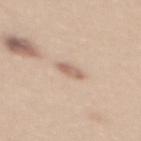Part of a total-body skin-imaging series; this lesion was reviewed on a skin check and was not flagged for biopsy.
On the upper back.
Captured under white-light illumination.
A female patient aged approximately 35.
A 15 mm close-up extracted from a 3D total-body photography capture.
The lesion's longest dimension is about 2.5 mm.